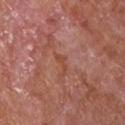Q: Is there a histopathology result?
A: catalogued during a skin exam; not biopsied
Q: What did automated image analysis measure?
A: a lesion area of about 2 mm², an outline eccentricity of about 0.9 (0 = round, 1 = elongated), and two-axis asymmetry of about 0.7
Q: Lesion size?
A: ~2.5 mm (longest diameter)
Q: Patient demographics?
A: male, roughly 65 years of age
Q: What kind of image is this?
A: ~15 mm tile from a whole-body skin photo
Q: What is the anatomic site?
A: the chest
Q: What lighting was used for the tile?
A: white-light illumination